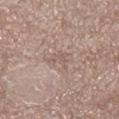biopsy status = total-body-photography surveillance lesion; no biopsy
subject = male, aged 48 to 52
image = total-body-photography crop, ~15 mm field of view
anatomic site = the left lower leg
automated metrics = a border-irregularity index near 6/10 and a peripheral color-asymmetry measure near 0.5
lesion size = ≈3 mm